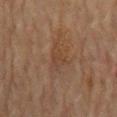Notes:
- follow-up — catalogued during a skin exam; not biopsied
- image — ~15 mm tile from a whole-body skin photo
- patient — female, aged 78–82
- diameter — about 3 mm
- anatomic site — the mid back